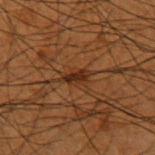biopsy status: catalogued during a skin exam; not biopsied
patient: male, approximately 50 years of age
lesion size: ~3 mm (longest diameter)
image: ~15 mm crop, total-body skin-cancer survey
tile lighting: cross-polarized illumination
site: the arm
automated lesion analysis: a footprint of about 3.5 mm², an outline eccentricity of about 0.9 (0 = round, 1 = elongated), and a shape-asymmetry score of about 0.3 (0 = symmetric); an average lesion color of about L≈23 a*≈17 b*≈25 (CIELAB) and a lesion–skin lightness drop of about 8; a color-variation rating of about 3/10 and radial color variation of about 1; an automated nevus-likeness rating near 65 out of 100 and a detector confidence of about 70 out of 100 that the crop contains a lesion